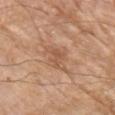| field | value |
|---|---|
| biopsy status | no biopsy performed (imaged during a skin exam) |
| location | the left forearm |
| patient | male, in their 80s |
| image | ~15 mm crop, total-body skin-cancer survey |
| lesion size | ~3 mm (longest diameter) |
| automated metrics | a mean CIELAB color near L≈54 a*≈22 b*≈33, a lesion–skin lightness drop of about 8, and a normalized border contrast of about 5.5; a border-irregularity index near 5/10, a within-lesion color-variation index near 0.5/10, and a peripheral color-asymmetry measure near 0 |